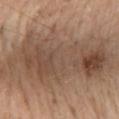Case summary:
– biopsy status · no biopsy performed (imaged during a skin exam)
– tile lighting · white-light
– automated lesion analysis · internal color variation of about 6.5 on a 0–10 scale and a peripheral color-asymmetry measure near 2.5; a classifier nevus-likeness of about 15/100
– imaging modality · ~15 mm tile from a whole-body skin photo
– site · the mid back
– subject · female, in their mid- to late 60s
– lesion size · about 13.5 mm